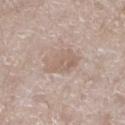Impression: Captured during whole-body skin photography for melanoma surveillance; the lesion was not biopsied. Context: From the left lower leg. Approximately 4.5 mm at its widest. A 15 mm close-up tile from a total-body photography series done for melanoma screening. A female patient aged 73–77.